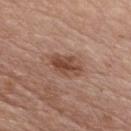Assessment: The lesion was photographed on a routine skin check and not biopsied; there is no pathology result. Context: From the chest. A male subject roughly 65 years of age. A 15 mm close-up tile from a total-body photography series done for melanoma screening. Captured under white-light illumination. The lesion-visualizer software estimated an area of roughly 9.5 mm², a shape eccentricity near 0.8, and a symmetry-axis asymmetry near 0.2. The analysis additionally found a mean CIELAB color near L≈48 a*≈21 b*≈28, a lesion–skin lightness drop of about 10, and a lesion-to-skin contrast of about 7.5 (normalized; higher = more distinct). The software also gave a classifier nevus-likeness of about 85/100 and a detector confidence of about 100 out of 100 that the crop contains a lesion. The recorded lesion diameter is about 4.5 mm.